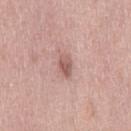Context: A roughly 15 mm field-of-view crop from a total-body skin photograph. The patient is a female aged approximately 40. Located on the right thigh.